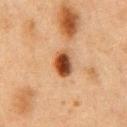Assessment: Recorded during total-body skin imaging; not selected for excision or biopsy. Context: Captured under cross-polarized illumination. The lesion's longest dimension is about 3.5 mm. A male subject aged 73–77. A 15 mm close-up extracted from a 3D total-body photography capture. The lesion is on the chest. Automated tile analysis of the lesion measured about 16 CIELAB-L* units darker than the surrounding skin and a lesion-to-skin contrast of about 12.5 (normalized; higher = more distinct). The analysis additionally found border irregularity of about 1.5 on a 0–10 scale, internal color variation of about 8.5 on a 0–10 scale, and a peripheral color-asymmetry measure near 2.5.